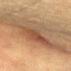Acquisition and patient details:
A male subject, roughly 85 years of age or older. Imaged with cross-polarized lighting. Approximately 4 mm at its widest. Automated image analysis of the tile measured a lesion area of about 10 mm², an eccentricity of roughly 0.7, and a shape-asymmetry score of about 0.25 (0 = symmetric). And it measured a border-irregularity rating of about 3/10. And it measured an automated nevus-likeness rating near 90 out of 100 and lesion-presence confidence of about 90/100. Located on the abdomen. Cropped from a total-body skin-imaging series; the visible field is about 15 mm.